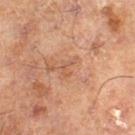{"biopsy_status": "not biopsied; imaged during a skin examination", "automated_metrics": {"area_mm2_approx": 2.5, "shape_asymmetry": 0.55, "border_irregularity_0_10": 6.5, "nevus_likeness_0_100": 0, "lesion_detection_confidence_0_100": 100}, "site": "left thigh", "patient": {"sex": "male", "age_approx": 70}, "lesion_size": {"long_diameter_mm_approx": 3.0}, "image": {"source": "total-body photography crop", "field_of_view_mm": 15}}